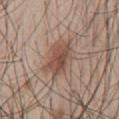The lesion is on the front of the torso. Longest diameter approximately 5 mm. The subject is a male in their mid-30s. The lesion-visualizer software estimated an average lesion color of about L≈50 a*≈19 b*≈26 (CIELAB) and roughly 11 lightness units darker than nearby skin. The tile uses white-light illumination. A 15 mm crop from a total-body photograph taken for skin-cancer surveillance.